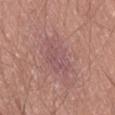Q: Is there a histopathology result?
A: total-body-photography surveillance lesion; no biopsy